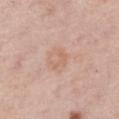biopsy_status: not biopsied; imaged during a skin examination
lighting: white-light
site: left lower leg
lesion_size:
  long_diameter_mm_approx: 2.5
patient:
  sex: female
  age_approx: 65
image:
  source: total-body photography crop
  field_of_view_mm: 15